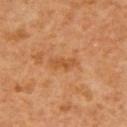Q: Is there a histopathology result?
A: total-body-photography surveillance lesion; no biopsy
Q: Who is the patient?
A: male, aged approximately 60
Q: Automated lesion metrics?
A: an area of roughly 4.5 mm², an eccentricity of roughly 0.9, and two-axis asymmetry of about 0.3; a lesion color around L≈52 a*≈25 b*≈40 in CIELAB; a detector confidence of about 100 out of 100 that the crop contains a lesion
Q: What is the anatomic site?
A: the right upper arm
Q: What lighting was used for the tile?
A: cross-polarized
Q: How was this image acquired?
A: total-body-photography crop, ~15 mm field of view
Q: What is the lesion's diameter?
A: ≈3.5 mm